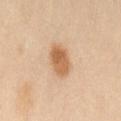Q: What are the patient's age and sex?
A: female, aged approximately 50
Q: What kind of image is this?
A: ~15 mm crop, total-body skin-cancer survey
Q: What is the anatomic site?
A: the lower back